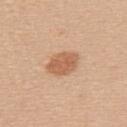diameter: ~3.5 mm (longest diameter); image source: 15 mm crop, total-body photography; site: the upper back; subject: female, about 40 years old; illumination: white-light.